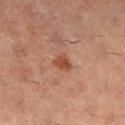Notes:
* biopsy status — catalogued during a skin exam; not biopsied
* tile lighting — cross-polarized illumination
* lesion diameter — ~2.5 mm (longest diameter)
* subject — female, aged 58 to 62
* imaging modality — 15 mm crop, total-body photography
* location — the right lower leg